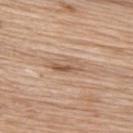notes: imaged on a skin check; not biopsied | image: 15 mm crop, total-body photography | location: the upper back | patient: female, roughly 65 years of age.